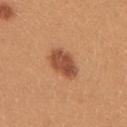workup = catalogued during a skin exam; not biopsied
lighting = white-light illumination
subject = female, about 25 years old
size = about 4 mm
anatomic site = the right upper arm
TBP lesion metrics = an automated nevus-likeness rating near 95 out of 100 and lesion-presence confidence of about 100/100
acquisition = ~15 mm crop, total-body skin-cancer survey